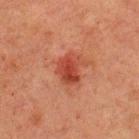notes = catalogued during a skin exam; not biopsied
site = the upper back
patient = male, about 60 years old
image = ~15 mm crop, total-body skin-cancer survey